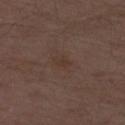{"biopsy_status": "not biopsied; imaged during a skin examination", "patient": {"sex": "male", "age_approx": 70}, "site": "left thigh", "image": {"source": "total-body photography crop", "field_of_view_mm": 15}}